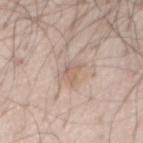  lighting: white-light
  image:
    source: total-body photography crop
    field_of_view_mm: 15
  patient:
    sex: male
    age_approx: 60
  site: abdomen
  lesion_size:
    long_diameter_mm_approx: 2.5